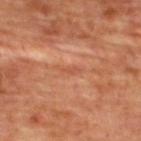Captured during whole-body skin photography for melanoma surveillance; the lesion was not biopsied. On the upper back. A roughly 15 mm field-of-view crop from a total-body skin photograph. The subject is a female aged approximately 45.